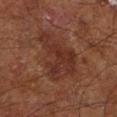Findings:
– biopsy status: total-body-photography surveillance lesion; no biopsy
– patient: male, aged 63 to 67
– tile lighting: cross-polarized illumination
– automated lesion analysis: a footprint of about 17 mm², an outline eccentricity of about 0.75 (0 = round, 1 = elongated), and two-axis asymmetry of about 0.6
– body site: the left lower leg
– image: 15 mm crop, total-body photography
– lesion size: ~6 mm (longest diameter)